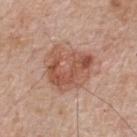{"biopsy_status": "not biopsied; imaged during a skin examination", "site": "upper back", "patient": {"sex": "male", "age_approx": 65}, "automated_metrics": {"area_mm2_approx": 23.0, "eccentricity": 0.5, "shape_asymmetry": 0.25, "vs_skin_darker_L": 10.0, "vs_skin_contrast_norm": 7.0, "border_irregularity_0_10": 2.5, "color_variation_0_10": 6.5, "peripheral_color_asymmetry": 2.5, "lesion_detection_confidence_0_100": 100}, "image": {"source": "total-body photography crop", "field_of_view_mm": 15}, "lesion_size": {"long_diameter_mm_approx": 5.5}, "lighting": "white-light"}